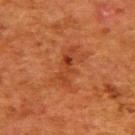Impression:
The lesion was tiled from a total-body skin photograph and was not biopsied.
Background:
Cropped from a total-body skin-imaging series; the visible field is about 15 mm. A female subject aged 48 to 52. On the upper back. The tile uses cross-polarized illumination. Longest diameter approximately 4.5 mm. Automated tile analysis of the lesion measured a shape-asymmetry score of about 0.35 (0 = symmetric).